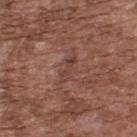{
  "patient": {
    "sex": "male",
    "age_approx": 75
  },
  "lesion_size": {
    "long_diameter_mm_approx": 3.0
  },
  "site": "back",
  "image": {
    "source": "total-body photography crop",
    "field_of_view_mm": 15
  },
  "lighting": "white-light"
}